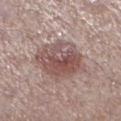Assessment:
The lesion was tiled from a total-body skin photograph and was not biopsied.
Context:
The total-body-photography lesion software estimated a lesion area of about 23 mm², a shape eccentricity near 0.55, and a shape-asymmetry score of about 0.2 (0 = symmetric). The analysis additionally found a lesion color around L≈52 a*≈18 b*≈20 in CIELAB and a lesion–skin lightness drop of about 11. The software also gave border irregularity of about 2 on a 0–10 scale and radial color variation of about 2.5. A male subject approximately 70 years of age. The lesion's longest dimension is about 6 mm. Located on the left lower leg. A 15 mm close-up tile from a total-body photography series done for melanoma screening. Imaged with white-light lighting.